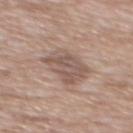Clinical impression:
Captured during whole-body skin photography for melanoma surveillance; the lesion was not biopsied.
Image and clinical context:
Longest diameter approximately 4 mm. A close-up tile cropped from a whole-body skin photograph, about 15 mm across. From the front of the torso. A male subject aged around 60.